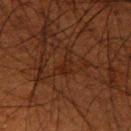No biopsy was performed on this lesion — it was imaged during a full skin examination and was not determined to be concerning. The patient is a male about 50 years old. The lesion is located on the right upper arm. Cropped from a whole-body photographic skin survey; the tile spans about 15 mm.